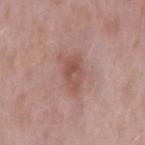workup = no biopsy performed (imaged during a skin exam); patient = male, in their mid- to late 50s; acquisition = ~15 mm tile from a whole-body skin photo; anatomic site = the back.